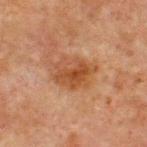biopsy status = catalogued during a skin exam; not biopsied
location = the chest
illumination = cross-polarized
image source = ~15 mm crop, total-body skin-cancer survey
patient = male, aged 63–67